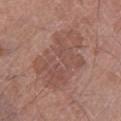Assessment: No biopsy was performed on this lesion — it was imaged during a full skin examination and was not determined to be concerning. Background: From the left lower leg. Captured under white-light illumination. Measured at roughly 8 mm in maximum diameter. A region of skin cropped from a whole-body photographic capture, roughly 15 mm wide. A male patient, aged approximately 60.